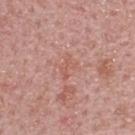biopsy_status: not biopsied; imaged during a skin examination
site: upper back
patient:
  sex: male
  age_approx: 50
image:
  source: total-body photography crop
  field_of_view_mm: 15
lesion_size:
  long_diameter_mm_approx: 3.0
automated_metrics:
  area_mm2_approx: 3.0
  border_irregularity_0_10: 6.0
  peripheral_color_asymmetry: 0.0
lighting: white-light